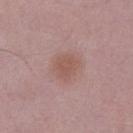{
  "biopsy_status": "not biopsied; imaged during a skin examination",
  "image": {
    "source": "total-body photography crop",
    "field_of_view_mm": 15
  },
  "lesion_size": {
    "long_diameter_mm_approx": 3.0
  },
  "lighting": "white-light",
  "patient": {
    "sex": "male",
    "age_approx": 50
  },
  "automated_metrics": {
    "area_mm2_approx": 7.0,
    "shape_asymmetry": 0.2,
    "peripheral_color_asymmetry": 0.5,
    "lesion_detection_confidence_0_100": 100
  },
  "site": "leg"
}